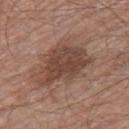Impression: This lesion was catalogued during total-body skin photography and was not selected for biopsy. Acquisition and patient details: Captured under white-light illumination. An algorithmic analysis of the crop reported a border-irregularity rating of about 3.5/10, a color-variation rating of about 3/10, and radial color variation of about 1. Located on the right thigh. The recorded lesion diameter is about 6.5 mm. A 15 mm close-up extracted from a 3D total-body photography capture. The patient is a male in their 60s.